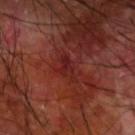Notes:
- workup — imaged on a skin check; not biopsied
- location — the arm
- image — ~15 mm crop, total-body skin-cancer survey
- patient — male, aged 58 to 62
- lesion size — ~3.5 mm (longest diameter)
- lighting — cross-polarized illumination